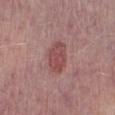{
  "biopsy_status": "not biopsied; imaged during a skin examination",
  "site": "right lower leg",
  "lighting": "white-light",
  "patient": {
    "sex": "male",
    "age_approx": 75
  },
  "image": {
    "source": "total-body photography crop",
    "field_of_view_mm": 15
  },
  "automated_metrics": {
    "cielab_L": 48,
    "cielab_a": 26,
    "cielab_b": 21,
    "vs_skin_contrast_norm": 7.0,
    "nevus_likeness_0_100": 90
  }
}